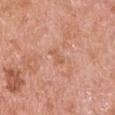Assessment:
This lesion was catalogued during total-body skin photography and was not selected for biopsy.
Background:
The lesion-visualizer software estimated a border-irregularity rating of about 4.5/10, a color-variation rating of about 0/10, and radial color variation of about 0. A male patient aged 68 to 72. A 15 mm close-up tile from a total-body photography series done for melanoma screening. Captured under white-light illumination. Located on the arm.